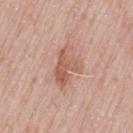* biopsy status · total-body-photography surveillance lesion; no biopsy
* image-analysis metrics · an area of roughly 10 mm² and an eccentricity of roughly 0.75; a mean CIELAB color near L≈58 a*≈22 b*≈29, roughly 9 lightness units darker than nearby skin, and a normalized border contrast of about 6.5; a peripheral color-asymmetry measure near 2; a lesion-detection confidence of about 100/100
* lesion size · ≈5 mm
* subject · female, roughly 60 years of age
* tile lighting · white-light
* site · the left thigh
* acquisition · 15 mm crop, total-body photography